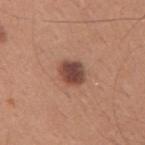The lesion was photographed on a routine skin check and not biopsied; there is no pathology result.
Located on the arm.
A male patient, aged 28 to 32.
A roughly 15 mm field-of-view crop from a total-body skin photograph.
Longest diameter approximately 3 mm.